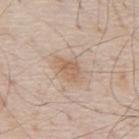Captured under white-light illumination.
About 3.5 mm across.
A region of skin cropped from a whole-body photographic capture, roughly 15 mm wide.
From the mid back.
A male subject, about 80 years old.